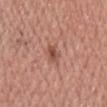follow-up: catalogued during a skin exam; not biopsied | diameter: ≈2.5 mm | subject: male, aged around 50 | body site: the chest | lighting: white-light | imaging modality: ~15 mm tile from a whole-body skin photo.